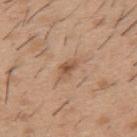Assessment: Recorded during total-body skin imaging; not selected for excision or biopsy. Acquisition and patient details: Approximately 2.5 mm at its widest. On the back. A male patient roughly 40 years of age. A 15 mm close-up extracted from a 3D total-body photography capture.